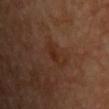Clinical impression: Imaged during a routine full-body skin examination; the lesion was not biopsied and no histopathology is available. Image and clinical context: A male subject aged 58 to 62. The lesion is on the upper back. A 15 mm crop from a total-body photograph taken for skin-cancer surveillance.